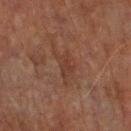Captured during whole-body skin photography for melanoma surveillance; the lesion was not biopsied. The patient is a male aged around 75. On the arm. This image is a 15 mm lesion crop taken from a total-body photograph. Captured under cross-polarized illumination. The lesion-visualizer software estimated a footprint of about 7.5 mm² and a symmetry-axis asymmetry near 0.5. And it measured a detector confidence of about 95 out of 100 that the crop contains a lesion. About 5 mm across.